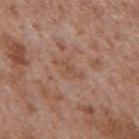Q: Where on the body is the lesion?
A: the back
Q: How was this image acquired?
A: 15 mm crop, total-body photography
Q: Who is the patient?
A: male, aged 63–67